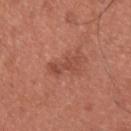Q: Was a biopsy performed?
A: imaged on a skin check; not biopsied
Q: Automated lesion metrics?
A: a footprint of about 4.5 mm², an outline eccentricity of about 0.9 (0 = round, 1 = elongated), and a symmetry-axis asymmetry near 0.35
Q: How large is the lesion?
A: ≈3.5 mm
Q: Patient demographics?
A: male, about 35 years old
Q: How was this image acquired?
A: ~15 mm tile from a whole-body skin photo
Q: What lighting was used for the tile?
A: white-light
Q: What is the anatomic site?
A: the back